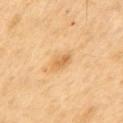Notes:
* workup: total-body-photography surveillance lesion; no biopsy
* lighting: cross-polarized
* size: about 3 mm
* anatomic site: the chest
* imaging modality: 15 mm crop, total-body photography
* patient: male, in their 70s
* TBP lesion metrics: a nevus-likeness score of about 15/100 and lesion-presence confidence of about 100/100